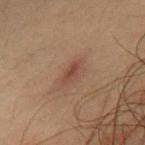Impression: Imaged during a routine full-body skin examination; the lesion was not biopsied and no histopathology is available. Image and clinical context: Longest diameter approximately 3 mm. The lesion is located on the chest. The subject is a male aged 48 to 52. A lesion tile, about 15 mm wide, cut from a 3D total-body photograph. Captured under cross-polarized illumination.